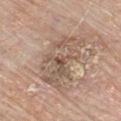{"biopsy_status": "not biopsied; imaged during a skin examination", "automated_metrics": {"color_variation_0_10": 6.0, "peripheral_color_asymmetry": 2.0, "nevus_likeness_0_100": 0, "lesion_detection_confidence_0_100": 65}, "site": "chest", "image": {"source": "total-body photography crop", "field_of_view_mm": 15}, "patient": {"sex": "male", "age_approx": 80}, "lesion_size": {"long_diameter_mm_approx": 7.5}}